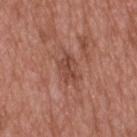<tbp_lesion>
  <biopsy_status>not biopsied; imaged during a skin examination</biopsy_status>
  <lesion_size>
    <long_diameter_mm_approx>3.5</long_diameter_mm_approx>
  </lesion_size>
  <site>head or neck</site>
  <image>
    <source>total-body photography crop</source>
    <field_of_view_mm>15</field_of_view_mm>
  </image>
  <patient>
    <sex>male</sex>
    <age_approx>50</age_approx>
  </patient>
  <lighting>white-light</lighting>
</tbp_lesion>